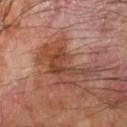{"biopsy_status": "not biopsied; imaged during a skin examination", "site": "left forearm", "lighting": "cross-polarized", "lesion_size": {"long_diameter_mm_approx": 7.0}, "automated_metrics": {"vs_skin_darker_L": 9.0, "vs_skin_contrast_norm": 7.5, "nevus_likeness_0_100": 0, "lesion_detection_confidence_0_100": 100}, "image": {"source": "total-body photography crop", "field_of_view_mm": 15}, "patient": {"sex": "male", "age_approx": 70}}